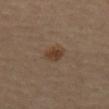Findings:
– workup · catalogued during a skin exam; not biopsied
– diameter · ~2.5 mm (longest diameter)
– automated metrics · an average lesion color of about L≈40 a*≈16 b*≈29 (CIELAB), about 8 CIELAB-L* units darker than the surrounding skin, and a normalized lesion–skin contrast near 8; a nevus-likeness score of about 90/100 and a lesion-detection confidence of about 100/100
– imaging modality · 15 mm crop, total-body photography
– lighting · cross-polarized
– patient · female, aged approximately 55
– body site · the chest